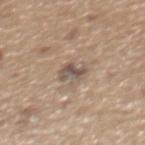Q: Was a biopsy performed?
A: no biopsy performed (imaged during a skin exam)
Q: What is the anatomic site?
A: the back
Q: What kind of image is this?
A: 15 mm crop, total-body photography
Q: What did automated image analysis measure?
A: a footprint of about 5 mm², an outline eccentricity of about 0.8 (0 = round, 1 = elongated), and a shape-asymmetry score of about 0.25 (0 = symmetric); a classifier nevus-likeness of about 0/100
Q: Who is the patient?
A: male, in their mid-60s
Q: What is the lesion's diameter?
A: ~3 mm (longest diameter)
Q: How was the tile lit?
A: white-light illumination